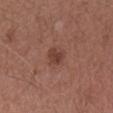Located on the right forearm. A roughly 15 mm field-of-view crop from a total-body skin photograph. A female subject, about 45 years old.